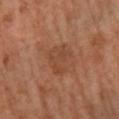{
  "biopsy_status": "not biopsied; imaged during a skin examination",
  "automated_metrics": {
    "area_mm2_approx": 8.0,
    "eccentricity": 0.75,
    "shape_asymmetry": 0.3
  },
  "site": "right lower leg",
  "patient": {
    "sex": "female",
    "age_approx": 65
  },
  "image": {
    "source": "total-body photography crop",
    "field_of_view_mm": 15
  }
}